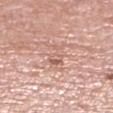The lesion was photographed on a routine skin check and not biopsied; there is no pathology result.